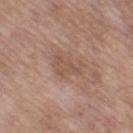notes=total-body-photography surveillance lesion; no biopsy | automated lesion analysis=an average lesion color of about L≈53 a*≈19 b*≈27 (CIELAB), about 7 CIELAB-L* units darker than the surrounding skin, and a normalized border contrast of about 5; a border-irregularity rating of about 5/10, a within-lesion color-variation index near 2/10, and a peripheral color-asymmetry measure near 0.5 | patient=female, aged 53 to 57 | image source=total-body-photography crop, ~15 mm field of view | body site=the leg | lesion size=≈4 mm.